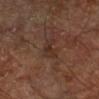This lesion was catalogued during total-body skin photography and was not selected for biopsy. A subject approximately 65 years of age. Cropped from a total-body skin-imaging series; the visible field is about 15 mm. On the right lower leg. The lesion's longest dimension is about 3.5 mm.